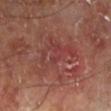follow-up — catalogued during a skin exam; not biopsied
patient — male, about 70 years old
anatomic site — the left lower leg
image source — total-body-photography crop, ~15 mm field of view